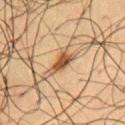Assessment:
Imaged during a routine full-body skin examination; the lesion was not biopsied and no histopathology is available.
Background:
About 3.5 mm across. Captured under cross-polarized illumination. A region of skin cropped from a whole-body photographic capture, roughly 15 mm wide. On the chest. A male subject aged around 60. Automated image analysis of the tile measured a lesion area of about 4.5 mm², a shape eccentricity near 0.9, and a shape-asymmetry score of about 0.35 (0 = symmetric). And it measured a mean CIELAB color near L≈40 a*≈17 b*≈30, roughly 13 lightness units darker than nearby skin, and a normalized lesion–skin contrast near 10.5. The software also gave a border-irregularity rating of about 3.5/10 and a peripheral color-asymmetry measure near 0.5. The analysis additionally found an automated nevus-likeness rating near 90 out of 100 and lesion-presence confidence of about 100/100.